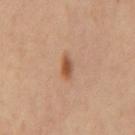Part of a total-body skin-imaging series; this lesion was reviewed on a skin check and was not flagged for biopsy. Located on the back. Cropped from a whole-body photographic skin survey; the tile spans about 15 mm. A male patient roughly 60 years of age. Imaged with cross-polarized lighting. Measured at roughly 2.5 mm in maximum diameter.